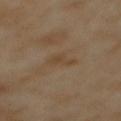| field | value |
|---|---|
| notes | no biopsy performed (imaged during a skin exam) |
| body site | the mid back |
| lesion size | about 3 mm |
| patient | female, about 55 years old |
| image | ~15 mm crop, total-body skin-cancer survey |